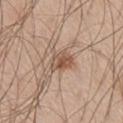Clinical impression: Part of a total-body skin-imaging series; this lesion was reviewed on a skin check and was not flagged for biopsy. Clinical summary: A close-up tile cropped from a whole-body skin photograph, about 15 mm across. The lesion's longest dimension is about 3.5 mm. Captured under white-light illumination. A male patient aged 58–62. The lesion is located on the right thigh.